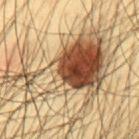No biopsy was performed on this lesion — it was imaged during a full skin examination and was not determined to be concerning. The patient is a male roughly 45 years of age. Imaged with cross-polarized lighting. The lesion's longest dimension is about 12 mm. On the mid back. This image is a 15 mm lesion crop taken from a total-body photograph.